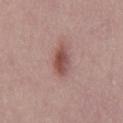- image-analysis metrics — an area of roughly 6.5 mm², an outline eccentricity of about 0.85 (0 = round, 1 = elongated), and two-axis asymmetry of about 0.25; a border-irregularity index near 2/10, internal color variation of about 4.5 on a 0–10 scale, and a peripheral color-asymmetry measure near 1.5; an automated nevus-likeness rating near 85 out of 100 and a lesion-detection confidence of about 100/100
- subject — male, approximately 30 years of age
- image source — ~15 mm tile from a whole-body skin photo
- lighting — white-light illumination